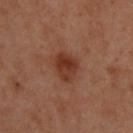image-analysis metrics: a footprint of about 7 mm² and an eccentricity of roughly 0.5; a classifier nevus-likeness of about 80/100 and a detector confidence of about 100 out of 100 that the crop contains a lesion
subject: male, aged approximately 40
site: the upper back
imaging modality: 15 mm crop, total-body photography
tile lighting: cross-polarized